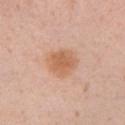Findings:
– notes — imaged on a skin check; not biopsied
– subject — female, roughly 35 years of age
– anatomic site — the left upper arm
– image source — ~15 mm crop, total-body skin-cancer survey